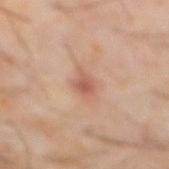anatomic site = the front of the torso; lighting = cross-polarized illumination; automated lesion analysis = lesion-presence confidence of about 100/100; subject = male, roughly 60 years of age; image = ~15 mm tile from a whole-body skin photo; lesion size = about 3 mm.